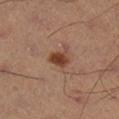Impression:
This lesion was catalogued during total-body skin photography and was not selected for biopsy.
Acquisition and patient details:
A lesion tile, about 15 mm wide, cut from a 3D total-body photograph. On the left lower leg. Automated image analysis of the tile measured a lesion area of about 4 mm², a shape eccentricity near 0.7, and a symmetry-axis asymmetry near 0.2. And it measured a mean CIELAB color near L≈34 a*≈19 b*≈26, a lesion–skin lightness drop of about 10, and a lesion-to-skin contrast of about 10 (normalized; higher = more distinct). The software also gave a border-irregularity index near 1.5/10, a color-variation rating of about 2.5/10, and radial color variation of about 1. It also reported a nevus-likeness score of about 95/100. A male subject, aged approximately 55.